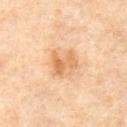| key | value |
|---|---|
| follow-up | catalogued during a skin exam; not biopsied |
| automated metrics | a lesion area of about 6.5 mm², an eccentricity of roughly 0.5, and a symmetry-axis asymmetry near 0.45; a mean CIELAB color near L≈67 a*≈23 b*≈40 and a normalized lesion–skin contrast near 6.5; a classifier nevus-likeness of about 35/100 and a detector confidence of about 100 out of 100 that the crop contains a lesion |
| lesion size | ≈3 mm |
| image source | total-body-photography crop, ~15 mm field of view |
| subject | female, roughly 50 years of age |
| illumination | cross-polarized |
| anatomic site | the left thigh |